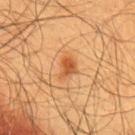Imaged during a routine full-body skin examination; the lesion was not biopsied and no histopathology is available. A 15 mm close-up extracted from a 3D total-body photography capture. Measured at roughly 2.5 mm in maximum diameter. Captured under cross-polarized illumination. The lesion is on the chest. Automated tile analysis of the lesion measured an average lesion color of about L≈52 a*≈26 b*≈40 (CIELAB), a lesion–skin lightness drop of about 11, and a normalized border contrast of about 7.5. A male subject approximately 60 years of age.